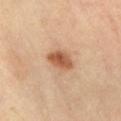A close-up tile cropped from a whole-body skin photograph, about 15 mm across. The tile uses cross-polarized illumination. The lesion-visualizer software estimated a footprint of about 6.5 mm², an eccentricity of roughly 0.75, and two-axis asymmetry of about 0.2. The analysis additionally found a lesion color around L≈54 a*≈22 b*≈34 in CIELAB and about 13 CIELAB-L* units darker than the surrounding skin. About 3.5 mm across. On the abdomen. A male subject, aged approximately 65.